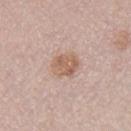workup = no biopsy performed (imaged during a skin exam); imaging modality = ~15 mm tile from a whole-body skin photo; lesion size = ≈3 mm; subject = male, aged 28 to 32; tile lighting = white-light.